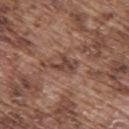Assessment: No biopsy was performed on this lesion — it was imaged during a full skin examination and was not determined to be concerning. Acquisition and patient details: The lesion is on the back. About 3 mm across. A 15 mm close-up tile from a total-body photography series done for melanoma screening. A male subject, roughly 75 years of age. Imaged with white-light lighting.